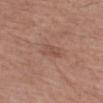Clinical impression: This lesion was catalogued during total-body skin photography and was not selected for biopsy.